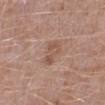Part of a total-body skin-imaging series; this lesion was reviewed on a skin check and was not flagged for biopsy.
A male subject aged 48–52.
Longest diameter approximately 3.5 mm.
Cropped from a whole-body photographic skin survey; the tile spans about 15 mm.
Automated image analysis of the tile measured an average lesion color of about L≈53 a*≈20 b*≈27 (CIELAB), roughly 7 lightness units darker than nearby skin, and a lesion-to-skin contrast of about 5.5 (normalized; higher = more distinct). It also reported border irregularity of about 4 on a 0–10 scale, a within-lesion color-variation index near 3/10, and peripheral color asymmetry of about 1.
From the left lower leg.